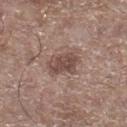This lesion was catalogued during total-body skin photography and was not selected for biopsy. A close-up tile cropped from a whole-body skin photograph, about 15 mm across. The tile uses white-light illumination. Located on the right lower leg. The patient is a male aged around 65.